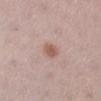workup: imaged on a skin check; not biopsied | acquisition: 15 mm crop, total-body photography | location: the right lower leg | patient: female, approximately 25 years of age | lesion size: about 2.5 mm | illumination: white-light.